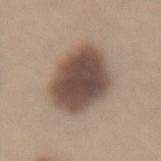Assessment: The lesion was tiled from a total-body skin photograph and was not biopsied. Acquisition and patient details: A female subject, aged around 40. The lesion is located on the lower back. A 15 mm crop from a total-body photograph taken for skin-cancer surveillance.